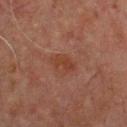Assessment:
The lesion was tiled from a total-body skin photograph and was not biopsied.
Image and clinical context:
A male subject, aged approximately 65. A 15 mm close-up tile from a total-body photography series done for melanoma screening. The lesion is on the upper back.